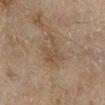Part of a total-body skin-imaging series; this lesion was reviewed on a skin check and was not flagged for biopsy.
The tile uses cross-polarized illumination.
The lesion is located on the right lower leg.
A lesion tile, about 15 mm wide, cut from a 3D total-body photograph.
A female patient roughly 60 years of age.